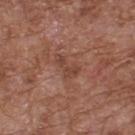  biopsy_status: not biopsied; imaged during a skin examination
  patient:
    sex: male
    age_approx: 75
  lesion_size:
    long_diameter_mm_approx: 3.5
  lighting: white-light
  site: upper back
  image:
    source: total-body photography crop
    field_of_view_mm: 15